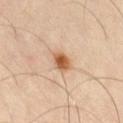Context:
A male patient approximately 65 years of age. Automated image analysis of the tile measured a lesion area of about 4.5 mm², a shape eccentricity near 0.45, and two-axis asymmetry of about 0.2. It also reported a detector confidence of about 100 out of 100 that the crop contains a lesion. Approximately 2.5 mm at its widest. Cropped from a whole-body photographic skin survey; the tile spans about 15 mm. Located on the right thigh.